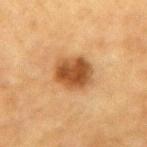workup: no biopsy performed (imaged during a skin exam); acquisition: total-body-photography crop, ~15 mm field of view; tile lighting: cross-polarized; site: the mid back; subject: male, aged 83 or over.